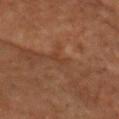{"biopsy_status": "not biopsied; imaged during a skin examination", "patient": {"sex": "male", "age_approx": 70}, "site": "head or neck", "image": {"source": "total-body photography crop", "field_of_view_mm": 15}, "automated_metrics": {"cielab_L": 29, "cielab_a": 17, "cielab_b": 24, "vs_skin_darker_L": 4.0, "vs_skin_contrast_norm": 4.5, "border_irregularity_0_10": 4.5, "color_variation_0_10": 0.0, "peripheral_color_asymmetry": 0.0}, "lesion_size": {"long_diameter_mm_approx": 2.5}, "lighting": "cross-polarized"}